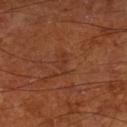| feature | finding |
|---|---|
| imaging modality | ~15 mm crop, total-body skin-cancer survey |
| patient | male, approximately 65 years of age |
| site | the leg |
| size | ≈3 mm |